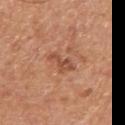Clinical impression: The lesion was tiled from a total-body skin photograph and was not biopsied. Clinical summary: The patient is a male aged approximately 65. Captured under white-light illumination. The total-body-photography lesion software estimated a lesion area of about 4 mm² and a shape-asymmetry score of about 0.55 (0 = symmetric). And it measured border irregularity of about 7 on a 0–10 scale, internal color variation of about 0.5 on a 0–10 scale, and a peripheral color-asymmetry measure near 0. And it measured a lesion-detection confidence of about 100/100. Cropped from a whole-body photographic skin survey; the tile spans about 15 mm. On the arm. The lesion's longest dimension is about 3.5 mm.